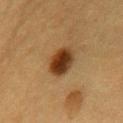The lesion was tiled from a total-body skin photograph and was not biopsied. The lesion is located on the chest. The patient is a female roughly 55 years of age. A 15 mm close-up extracted from a 3D total-body photography capture. The recorded lesion diameter is about 4 mm. Captured under cross-polarized illumination.